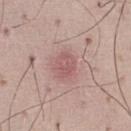Captured during whole-body skin photography for melanoma surveillance; the lesion was not biopsied. A male patient aged 48 to 52. The total-body-photography lesion software estimated a shape eccentricity near 0.65 and two-axis asymmetry of about 0.15. It also reported a border-irregularity index near 1.5/10, a color-variation rating of about 3/10, and peripheral color asymmetry of about 1. And it measured a detector confidence of about 100 out of 100 that the crop contains a lesion. A 15 mm close-up extracted from a 3D total-body photography capture. Imaged with white-light lighting. The lesion's longest dimension is about 3 mm. The lesion is on the left thigh.